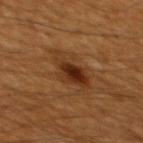workup: catalogued during a skin exam; not biopsied
site: the mid back
automated metrics: a mean CIELAB color near L≈30 a*≈20 b*≈31, roughly 9 lightness units darker than nearby skin, and a lesion-to-skin contrast of about 8.5 (normalized; higher = more distinct); border irregularity of about 4 on a 0–10 scale and peripheral color asymmetry of about 2; an automated nevus-likeness rating near 90 out of 100
subject: male, approximately 60 years of age
diameter: ~5.5 mm (longest diameter)
acquisition: total-body-photography crop, ~15 mm field of view
lighting: cross-polarized illumination